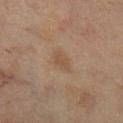<tbp_lesion>
<lighting>cross-polarized</lighting>
<patient>
  <sex>female</sex>
  <age_approx>55</age_approx>
</patient>
<site>right lower leg</site>
<image>
  <source>total-body photography crop</source>
  <field_of_view_mm>15</field_of_view_mm>
</image>
</tbp_lesion>